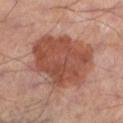<tbp_lesion>
  <biopsy_status>not biopsied; imaged during a skin examination</biopsy_status>
  <patient>
    <sex>male</sex>
    <age_approx>55</age_approx>
  </patient>
  <image>
    <source>total-body photography crop</source>
    <field_of_view_mm>15</field_of_view_mm>
  </image>
  <site>left lower leg</site>
</tbp_lesion>